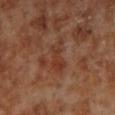Part of a total-body skin-imaging series; this lesion was reviewed on a skin check and was not flagged for biopsy. Captured under cross-polarized illumination. The lesion's longest dimension is about 4 mm. A 15 mm crop from a total-body photograph taken for skin-cancer surveillance. The lesion-visualizer software estimated a border-irregularity rating of about 4.5/10, internal color variation of about 3.5 on a 0–10 scale, and a peripheral color-asymmetry measure near 1. On the left lower leg. A male subject, approximately 70 years of age.